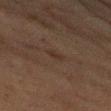Q: Is there a histopathology result?
A: imaged on a skin check; not biopsied
Q: What lighting was used for the tile?
A: cross-polarized
Q: How large is the lesion?
A: ~2.5 mm (longest diameter)
Q: Where on the body is the lesion?
A: the right upper arm
Q: What kind of image is this?
A: 15 mm crop, total-body photography
Q: Patient demographics?
A: male, approximately 75 years of age